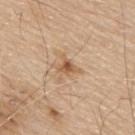The lesion was tiled from a total-body skin photograph and was not biopsied.
From the back.
A male subject aged around 80.
A 15 mm close-up extracted from a 3D total-body photography capture.
About 3.5 mm across.